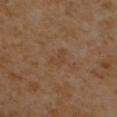notes: imaged on a skin check; not biopsied | anatomic site: the chest | lighting: cross-polarized | lesion diameter: about 3 mm | imaging modality: total-body-photography crop, ~15 mm field of view | subject: female, in their mid- to late 50s.